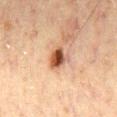biopsy status — no biopsy performed (imaged during a skin exam); size — about 3 mm; image-analysis metrics — a lesion area of about 6 mm², an outline eccentricity of about 0.55 (0 = round, 1 = elongated), and a symmetry-axis asymmetry near 0.25; subject — male, aged around 50; tile lighting — cross-polarized illumination; image source — ~15 mm crop, total-body skin-cancer survey; anatomic site — the mid back.